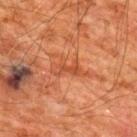Clinical impression: Recorded during total-body skin imaging; not selected for excision or biopsy. Acquisition and patient details: A male subject, aged around 60. About 4.5 mm across. Imaged with cross-polarized lighting. Automated tile analysis of the lesion measured roughly 6 lightness units darker than nearby skin and a lesion-to-skin contrast of about 5.5 (normalized; higher = more distinct). The analysis additionally found internal color variation of about 3 on a 0–10 scale and a peripheral color-asymmetry measure near 1. Cropped from a whole-body photographic skin survey; the tile spans about 15 mm. The lesion is located on the upper back.